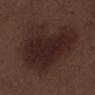Q: Was this lesion biopsied?
A: total-body-photography surveillance lesion; no biopsy
Q: Patient demographics?
A: male, approximately 70 years of age
Q: How was the tile lit?
A: white-light illumination
Q: Automated lesion metrics?
A: an area of roughly 50 mm² and an eccentricity of roughly 0.6; an average lesion color of about L≈23 a*≈17 b*≈17 (CIELAB) and a normalized border contrast of about 9; a classifier nevus-likeness of about 55/100
Q: Lesion location?
A: the left lower leg
Q: What is the imaging modality?
A: total-body-photography crop, ~15 mm field of view